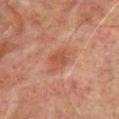Context:
The lesion's longest dimension is about 3.5 mm. This is a cross-polarized tile. Located on the front of the torso. A lesion tile, about 15 mm wide, cut from a 3D total-body photograph. Automated tile analysis of the lesion measured an area of roughly 5.5 mm², an outline eccentricity of about 0.75 (0 = round, 1 = elongated), and a symmetry-axis asymmetry near 0.2. The software also gave a mean CIELAB color near L≈41 a*≈22 b*≈26, roughly 7 lightness units darker than nearby skin, and a normalized lesion–skin contrast near 6. The analysis additionally found internal color variation of about 1.5 on a 0–10 scale and peripheral color asymmetry of about 0.5. It also reported an automated nevus-likeness rating near 50 out of 100 and a lesion-detection confidence of about 100/100. The subject is a male approximately 65 years of age.